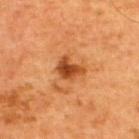Part of a total-body skin-imaging series; this lesion was reviewed on a skin check and was not flagged for biopsy. The lesion is on the upper back. The subject is a male about 65 years old. Measured at roughly 3 mm in maximum diameter. This is a cross-polarized tile. A 15 mm close-up tile from a total-body photography series done for melanoma screening.